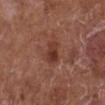biopsy status = no biopsy performed (imaged during a skin exam)
subject = male, in their mid- to late 70s
automated lesion analysis = a border-irregularity index near 3/10 and a within-lesion color-variation index near 4.5/10; a classifier nevus-likeness of about 55/100 and a detector confidence of about 100 out of 100 that the crop contains a lesion
anatomic site = the left lower leg
image = ~15 mm tile from a whole-body skin photo
diameter = ≈2.5 mm